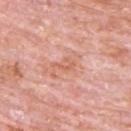Findings:
• follow-up: imaged on a skin check; not biopsied
• automated lesion analysis: a lesion area of about 3 mm² and a shape eccentricity near 0.85; a mean CIELAB color near L≈63 a*≈26 b*≈32, about 7 CIELAB-L* units darker than the surrounding skin, and a lesion-to-skin contrast of about 5.5 (normalized; higher = more distinct); a border-irregularity index near 4.5/10, internal color variation of about 2.5 on a 0–10 scale, and peripheral color asymmetry of about 1; an automated nevus-likeness rating near 0 out of 100 and a lesion-detection confidence of about 95/100
• lighting: white-light illumination
• location: the back
• acquisition: total-body-photography crop, ~15 mm field of view
• subject: male, aged around 80